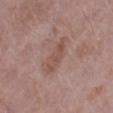• biopsy status · imaged on a skin check; not biopsied
• anatomic site · the right lower leg
• subject · female, roughly 70 years of age
• size · about 5 mm
• illumination · white-light illumination
• acquisition · 15 mm crop, total-body photography